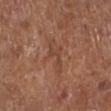{
  "biopsy_status": "not biopsied; imaged during a skin examination"
}